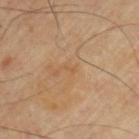* notes: total-body-photography surveillance lesion; no biopsy